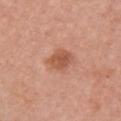Clinical summary: A female subject, roughly 60 years of age. The total-body-photography lesion software estimated a mean CIELAB color near L≈54 a*≈26 b*≈33 and a lesion–skin lightness drop of about 11. It also reported a classifier nevus-likeness of about 50/100 and a lesion-detection confidence of about 100/100. The lesion is located on the right upper arm. A region of skin cropped from a whole-body photographic capture, roughly 15 mm wide.